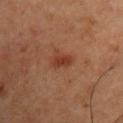No biopsy was performed on this lesion — it was imaged during a full skin examination and was not determined to be concerning. About 2.5 mm across. A lesion tile, about 15 mm wide, cut from a 3D total-body photograph. A male subject in their mid- to late 50s. The lesion is located on the front of the torso.